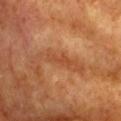The lesion was photographed on a routine skin check and not biopsied; there is no pathology result. On the chest. The total-body-photography lesion software estimated a lesion color around L≈49 a*≈28 b*≈39 in CIELAB, about 8 CIELAB-L* units darker than the surrounding skin, and a normalized lesion–skin contrast near 6. The analysis additionally found border irregularity of about 4 on a 0–10 scale and a peripheral color-asymmetry measure near 0. It also reported a nevus-likeness score of about 0/100 and lesion-presence confidence of about 70/100. A female subject, about 75 years old. Imaged with cross-polarized lighting. A 15 mm close-up tile from a total-body photography series done for melanoma screening.